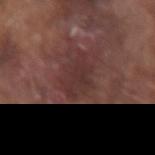The lesion was tiled from a total-body skin photograph and was not biopsied.
A 15 mm close-up extracted from a 3D total-body photography capture.
Located on the right forearm.
The lesion's longest dimension is about 4.5 mm.
A male patient, aged around 75.